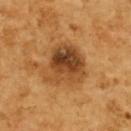This lesion was catalogued during total-body skin photography and was not selected for biopsy. A region of skin cropped from a whole-body photographic capture, roughly 15 mm wide. A female subject, in their mid-50s. The lesion is on the upper back.